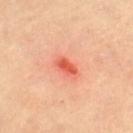Assessment: This lesion was catalogued during total-body skin photography and was not selected for biopsy. Context: From the right thigh. A 15 mm close-up tile from a total-body photography series done for melanoma screening. The patient is a female approximately 75 years of age. Approximately 2.5 mm at its widest. The lesion-visualizer software estimated an outline eccentricity of about 0.8 (0 = round, 1 = elongated) and two-axis asymmetry of about 0.35. And it measured an average lesion color of about L≈61 a*≈42 b*≈40 (CIELAB) and about 13 CIELAB-L* units darker than the surrounding skin.